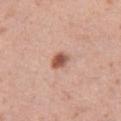workup: catalogued during a skin exam; not biopsied.